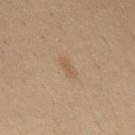Assessment:
The lesion was tiled from a total-body skin photograph and was not biopsied.
Acquisition and patient details:
Captured under cross-polarized illumination. A roughly 15 mm field-of-view crop from a total-body skin photograph. The subject is a female aged 48 to 52. Automated image analysis of the tile measured a lesion area of about 3.5 mm² and a symmetry-axis asymmetry near 0.3. It also reported an average lesion color of about L≈48 a*≈12 b*≈29 (CIELAB) and a lesion-to-skin contrast of about 5 (normalized; higher = more distinct). The analysis additionally found a border-irregularity index near 2.5/10, a color-variation rating of about 1/10, and a peripheral color-asymmetry measure near 0.5. The lesion's longest dimension is about 3 mm. Located on the mid back.